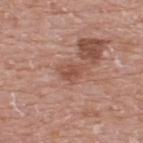  biopsy_status: not biopsied; imaged during a skin examination
  patient:
    sex: male
    age_approx: 75
  image:
    source: total-body photography crop
    field_of_view_mm: 15
  site: upper back